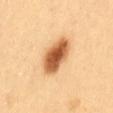follow-up: imaged on a skin check; not biopsied | lesion diameter: ~5 mm (longest diameter) | subject: female, in their mid- to late 50s | image: total-body-photography crop, ~15 mm field of view | body site: the abdomen.